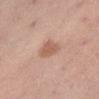* notes — catalogued during a skin exam; not biopsied
* subject — female, approximately 40 years of age
* anatomic site — the abdomen
* tile lighting — white-light
* image source — ~15 mm tile from a whole-body skin photo
* size — ≈3 mm